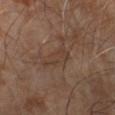No biopsy was performed on this lesion — it was imaged during a full skin examination and was not determined to be concerning.
The lesion is on the left forearm.
A male patient, approximately 65 years of age.
Approximately 4.5 mm at its widest.
A 15 mm crop from a total-body photograph taken for skin-cancer surveillance.
This is a cross-polarized tile.